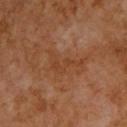Imaged during a routine full-body skin examination; the lesion was not biopsied and no histopathology is available. A male subject, approximately 60 years of age. The recorded lesion diameter is about 5 mm. Automated tile analysis of the lesion measured a footprint of about 7 mm² and a symmetry-axis asymmetry near 0.65. And it measured an average lesion color of about L≈40 a*≈22 b*≈33 (CIELAB) and about 5 CIELAB-L* units darker than the surrounding skin. A region of skin cropped from a whole-body photographic capture, roughly 15 mm wide. The lesion is located on the chest. Captured under cross-polarized illumination.